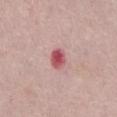notes = total-body-photography surveillance lesion; no biopsy
lesion size = about 2.5 mm
image-analysis metrics = an area of roughly 5 mm², a shape eccentricity near 0.6, and a symmetry-axis asymmetry near 0.2; a color-variation rating of about 5.5/10 and radial color variation of about 1.5
patient = female, in their mid-60s
illumination = white-light
imaging modality = ~15 mm tile from a whole-body skin photo
body site = the abdomen